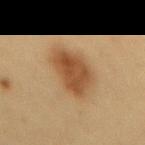Clinical impression:
The lesion was tiled from a total-body skin photograph and was not biopsied.